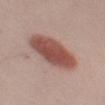Recorded during total-body skin imaging; not selected for excision or biopsy. Captured under white-light illumination. The patient is a male approximately 50 years of age. About 7 mm across. A region of skin cropped from a whole-body photographic capture, roughly 15 mm wide. The lesion is on the left upper arm.